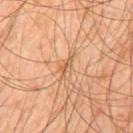Clinical impression: Captured during whole-body skin photography for melanoma surveillance; the lesion was not biopsied.